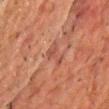Recorded during total-body skin imaging; not selected for excision or biopsy. Automated image analysis of the tile measured a lesion color around L≈38 a*≈21 b*≈24 in CIELAB, roughly 6 lightness units darker than nearby skin, and a lesion-to-skin contrast of about 5 (normalized; higher = more distinct). It also reported a lesion-detection confidence of about 90/100. A lesion tile, about 15 mm wide, cut from a 3D total-body photograph. Imaged with cross-polarized lighting. The patient is a male aged approximately 60. Approximately 3 mm at its widest. The lesion is on the chest.